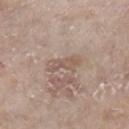Recorded during total-body skin imaging; not selected for excision or biopsy.